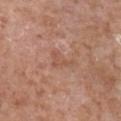Findings:
– biopsy status · total-body-photography surveillance lesion; no biopsy
– lighting · white-light
– image · ~15 mm tile from a whole-body skin photo
– anatomic site · the right lower leg
– subject · male, aged 73 to 77
– lesion diameter · ≈3 mm
– automated metrics · an average lesion color of about L≈53 a*≈22 b*≈30 (CIELAB), about 6 CIELAB-L* units darker than the surrounding skin, and a lesion-to-skin contrast of about 5 (normalized; higher = more distinct); a nevus-likeness score of about 0/100